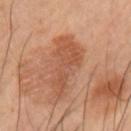Q: Was a biopsy performed?
A: no biopsy performed (imaged during a skin exam)
Q: What is the imaging modality?
A: ~15 mm crop, total-body skin-cancer survey
Q: How large is the lesion?
A: ≈7 mm
Q: What did automated image analysis measure?
A: a classifier nevus-likeness of about 0/100 and a lesion-detection confidence of about 100/100
Q: What lighting was used for the tile?
A: cross-polarized illumination
Q: Where on the body is the lesion?
A: the left upper arm
Q: Patient demographics?
A: male, in their 60s